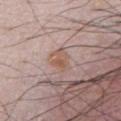Imaged during a routine full-body skin examination; the lesion was not biopsied and no histopathology is available. Located on the chest. A 15 mm crop from a total-body photograph taken for skin-cancer surveillance. The lesion's longest dimension is about 3 mm. The subject is a male aged 48–52.